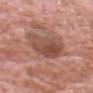biopsy status: catalogued during a skin exam; not biopsied
lighting: white-light
acquisition: 15 mm crop, total-body photography
subject: male, aged 78–82
location: the head or neck
lesion diameter: ≈5.5 mm